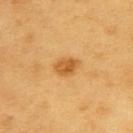Part of a total-body skin-imaging series; this lesion was reviewed on a skin check and was not flagged for biopsy. A roughly 15 mm field-of-view crop from a total-body skin photograph. The tile uses cross-polarized illumination. The lesion is located on the back. About 3 mm across. A male subject in their 60s.